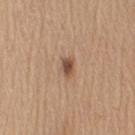Impression:
The lesion was photographed on a routine skin check and not biopsied; there is no pathology result.
Context:
A 15 mm close-up extracted from a 3D total-body photography capture. On the right upper arm. A male subject, in their mid- to late 60s.